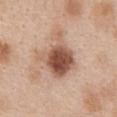Located on the abdomen. A region of skin cropped from a whole-body photographic capture, roughly 15 mm wide. The patient is a female roughly 40 years of age.